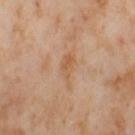Clinical impression: Recorded during total-body skin imaging; not selected for excision or biopsy. Context: Approximately 4 mm at its widest. Located on the left thigh. A female patient roughly 55 years of age. A lesion tile, about 15 mm wide, cut from a 3D total-body photograph. Automated image analysis of the tile measured about 7 CIELAB-L* units darker than the surrounding skin and a normalized lesion–skin contrast near 5.5. Imaged with cross-polarized lighting.